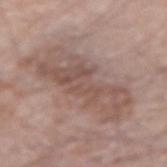notes = total-body-photography surveillance lesion; no biopsy | automated lesion analysis = a lesion color around L≈53 a*≈17 b*≈23 in CIELAB, roughly 8 lightness units darker than nearby skin, and a normalized border contrast of about 6 | acquisition = 15 mm crop, total-body photography | patient = male, in their 80s | tile lighting = white-light | site = the right arm | lesion diameter = about 10.5 mm.